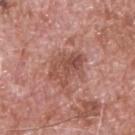patient: male, aged around 60
image-analysis metrics: an area of roughly 12 mm² and a symmetry-axis asymmetry near 0.3; a lesion color around L≈51 a*≈24 b*≈27 in CIELAB, roughly 9 lightness units darker than nearby skin, and a normalized lesion–skin contrast near 6.5
size: ≈4.5 mm
anatomic site: the upper back
imaging modality: ~15 mm tile from a whole-body skin photo